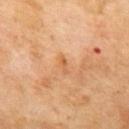Q: Was a biopsy performed?
A: total-body-photography surveillance lesion; no biopsy
Q: Who is the patient?
A: male, aged around 70
Q: How was this image acquired?
A: ~15 mm tile from a whole-body skin photo
Q: Illumination type?
A: cross-polarized
Q: What is the lesion's diameter?
A: ≈2.5 mm
Q: Where on the body is the lesion?
A: the back